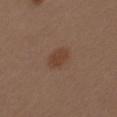biopsy status — total-body-photography surveillance lesion; no biopsy | patient — female, in their 40s | image — total-body-photography crop, ~15 mm field of view | size — ~3 mm (longest diameter) | illumination — white-light illumination | image-analysis metrics — a lesion area of about 5 mm² and a shape eccentricity near 0.75; a lesion–skin lightness drop of about 7 and a normalized border contrast of about 6.5; a classifier nevus-likeness of about 90/100 and lesion-presence confidence of about 100/100 | body site — the left upper arm.